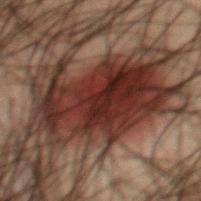Part of a total-body skin-imaging series; this lesion was reviewed on a skin check and was not flagged for biopsy. A male patient, roughly 50 years of age. From the mid back. Cropped from a whole-body photographic skin survey; the tile spans about 15 mm.